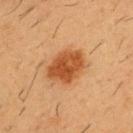Findings:
* image source · ~15 mm crop, total-body skin-cancer survey
* anatomic site · the chest
* size · ≈5 mm
* illumination · cross-polarized
* automated lesion analysis · a shape eccentricity near 0.7 and a symmetry-axis asymmetry near 0.2; an automated nevus-likeness rating near 100 out of 100
* patient · male, roughly 55 years of age